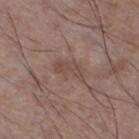Recorded during total-body skin imaging; not selected for excision or biopsy.
The tile uses white-light illumination.
Cropped from a whole-body photographic skin survey; the tile spans about 15 mm.
A male patient aged around 50.
The lesion is on the left lower leg.